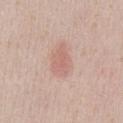biopsy_status: not biopsied; imaged during a skin examination
site: chest
patient:
  sex: male
  age_approx: 40
automated_metrics:
  eccentricity: 0.8
  shape_asymmetry: 0.2
  border_irregularity_0_10: 2.0
  color_variation_0_10: 2.5
  peripheral_color_asymmetry: 1.0
image:
  source: total-body photography crop
  field_of_view_mm: 15